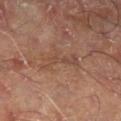No biopsy was performed on this lesion — it was imaged during a full skin examination and was not determined to be concerning. A male patient about 70 years old. A lesion tile, about 15 mm wide, cut from a 3D total-body photograph. Imaged with cross-polarized lighting. The lesion is on the right lower leg. Automated tile analysis of the lesion measured a footprint of about 7 mm², an outline eccentricity of about 0.95 (0 = round, 1 = elongated), and a shape-asymmetry score of about 0.45 (0 = symmetric). And it measured border irregularity of about 7 on a 0–10 scale, a within-lesion color-variation index near 2.5/10, and peripheral color asymmetry of about 0.5. The analysis additionally found lesion-presence confidence of about 75/100.